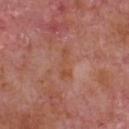biopsy status: total-body-photography surveillance lesion; no biopsy | image: ~15 mm crop, total-body skin-cancer survey | patient: male, in their mid-60s | body site: the chest.